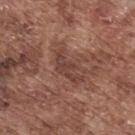  lesion_size:
    long_diameter_mm_approx: 4.5
  site: upper back
  patient:
    sex: male
    age_approx: 75
  image:
    source: total-body photography crop
    field_of_view_mm: 15
  automated_metrics:
    cielab_L: 42
    cielab_a: 21
    cielab_b: 24
    vs_skin_darker_L: 8.0
    vs_skin_contrast_norm: 6.5
    border_irregularity_0_10: 5.0
    color_variation_0_10: 2.5
    peripheral_color_asymmetry: 0.5